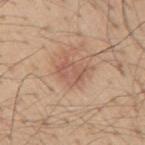| feature | finding |
|---|---|
| automated lesion analysis | an area of roughly 7 mm², a shape eccentricity near 0.7, and a symmetry-axis asymmetry near 0.35; an automated nevus-likeness rating near 55 out of 100 and a detector confidence of about 100 out of 100 that the crop contains a lesion |
| imaging modality | total-body-photography crop, ~15 mm field of view |
| subject | male, roughly 55 years of age |
| anatomic site | the right upper arm |